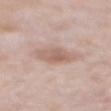The lesion was tiled from a total-body skin photograph and was not biopsied. Approximately 3.5 mm at its widest. The lesion is on the back. The tile uses white-light illumination. A male subject, aged around 75. A roughly 15 mm field-of-view crop from a total-body skin photograph.